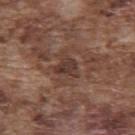Findings:
• biopsy status: catalogued during a skin exam; not biopsied
• patient: male, about 75 years old
• image source: total-body-photography crop, ~15 mm field of view
• anatomic site: the upper back